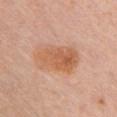{"biopsy_status": "not biopsied; imaged during a skin examination", "patient": {"sex": "female", "age_approx": 60}, "site": "chest", "lesion_size": {"long_diameter_mm_approx": 5.5}, "lighting": "white-light", "image": {"source": "total-body photography crop", "field_of_view_mm": 15}, "automated_metrics": {"area_mm2_approx": 15.0, "eccentricity": 0.8, "shape_asymmetry": 0.2, "cielab_L": 60, "cielab_a": 24, "cielab_b": 36, "vs_skin_darker_L": 9.0, "nevus_likeness_0_100": 95, "lesion_detection_confidence_0_100": 100}}